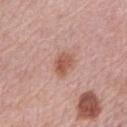workup: total-body-photography surveillance lesion; no biopsy
acquisition: 15 mm crop, total-body photography
TBP lesion metrics: an eccentricity of roughly 0.7 and a shape-asymmetry score of about 0.2 (0 = symmetric); a border-irregularity index near 2/10 and a peripheral color-asymmetry measure near 1; an automated nevus-likeness rating near 85 out of 100 and a lesion-detection confidence of about 100/100
location: the right upper arm
size: about 3 mm
subject: female, about 65 years old
illumination: white-light illumination